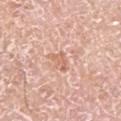Assessment:
Captured during whole-body skin photography for melanoma surveillance; the lesion was not biopsied.
Clinical summary:
Automated tile analysis of the lesion measured an automated nevus-likeness rating near 0 out of 100 and a detector confidence of about 100 out of 100 that the crop contains a lesion. The lesion is on the left upper arm. A male subject, aged around 75. The recorded lesion diameter is about 2.5 mm. A close-up tile cropped from a whole-body skin photograph, about 15 mm across. Imaged with white-light lighting.